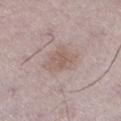notes=no biopsy performed (imaged during a skin exam) | anatomic site=the left lower leg | subject=male, about 50 years old | imaging modality=total-body-photography crop, ~15 mm field of view.